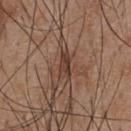| key | value |
|---|---|
| biopsy status | imaged on a skin check; not biopsied |
| image | total-body-photography crop, ~15 mm field of view |
| body site | the front of the torso |
| subject | male, aged 53–57 |